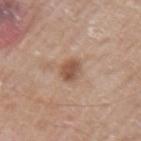Findings:
- workup — catalogued during a skin exam; not biopsied
- patient — male, in their 60s
- location — the arm
- image source — 15 mm crop, total-body photography
- automated lesion analysis — a lesion color around L≈53 a*≈20 b*≈29 in CIELAB, about 11 CIELAB-L* units darker than the surrounding skin, and a lesion-to-skin contrast of about 8 (normalized; higher = more distinct); a border-irregularity index near 2/10 and a peripheral color-asymmetry measure near 1
- lesion diameter — ~3 mm (longest diameter)
- illumination — white-light